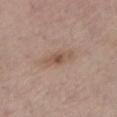About 4.5 mm across. Cropped from a whole-body photographic skin survey; the tile spans about 15 mm. Imaged with white-light lighting. The lesion is located on the left lower leg. A female patient, approximately 65 years of age.